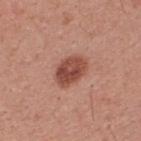This lesion was catalogued during total-body skin photography and was not selected for biopsy.
The lesion is located on the upper back.
The patient is a male aged 28 to 32.
A lesion tile, about 15 mm wide, cut from a 3D total-body photograph.